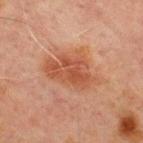Imaged during a routine full-body skin examination; the lesion was not biopsied and no histopathology is available. A male subject, aged 68 to 72. A 15 mm crop from a total-body photograph taken for skin-cancer surveillance. An algorithmic analysis of the crop reported a mean CIELAB color near L≈44 a*≈22 b*≈29, a lesion–skin lightness drop of about 8, and a lesion-to-skin contrast of about 7 (normalized; higher = more distinct). The lesion is on the front of the torso. The tile uses cross-polarized illumination. The lesion's longest dimension is about 7 mm.